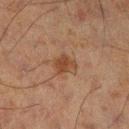Impression:
Captured during whole-body skin photography for melanoma surveillance; the lesion was not biopsied.
Clinical summary:
The lesion-visualizer software estimated about 7 CIELAB-L* units darker than the surrounding skin and a lesion-to-skin contrast of about 7.5 (normalized; higher = more distinct). And it measured a border-irregularity rating of about 3/10 and peripheral color asymmetry of about 0.5. A close-up tile cropped from a whole-body skin photograph, about 15 mm across. Located on the left leg. About 2.5 mm across. A male patient approximately 60 years of age.